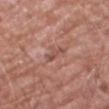The lesion was tiled from a total-body skin photograph and was not biopsied.
The lesion is on the left forearm.
Imaged with white-light lighting.
An algorithmic analysis of the crop reported a border-irregularity index near 4.5/10, internal color variation of about 0 on a 0–10 scale, and peripheral color asymmetry of about 0. It also reported an automated nevus-likeness rating near 0 out of 100 and a detector confidence of about 95 out of 100 that the crop contains a lesion.
A male patient aged around 60.
Approximately 3 mm at its widest.
A 15 mm close-up extracted from a 3D total-body photography capture.